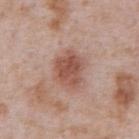Impression:
The lesion was photographed on a routine skin check and not biopsied; there is no pathology result.
Image and clinical context:
A male patient aged approximately 65. A 15 mm close-up extracted from a 3D total-body photography capture. The lesion's longest dimension is about 4 mm. From the abdomen.